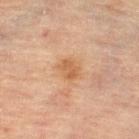Q: Was a biopsy performed?
A: catalogued during a skin exam; not biopsied
Q: What lighting was used for the tile?
A: cross-polarized
Q: Lesion location?
A: the left lower leg
Q: Lesion size?
A: ≈3 mm
Q: What is the imaging modality?
A: 15 mm crop, total-body photography
Q: What are the patient's age and sex?
A: female, roughly 80 years of age